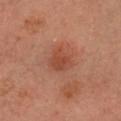No biopsy was performed on this lesion — it was imaged during a full skin examination and was not determined to be concerning.
The lesion is located on the head or neck.
Imaged with cross-polarized lighting.
A 15 mm close-up extracted from a 3D total-body photography capture.
The patient is a male aged around 55.